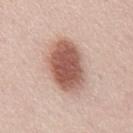– notes · catalogued during a skin exam; not biopsied
– subject · male, about 50 years old
– image-analysis metrics · an area of roughly 22 mm² and a shape-asymmetry score of about 0.1 (0 = symmetric); an average lesion color of about L≈56 a*≈22 b*≈27 (CIELAB); border irregularity of about 1.5 on a 0–10 scale, internal color variation of about 5 on a 0–10 scale, and radial color variation of about 1.5; a nevus-likeness score of about 100/100 and a detector confidence of about 100 out of 100 that the crop contains a lesion
– imaging modality · ~15 mm crop, total-body skin-cancer survey
– anatomic site · the abdomen
– illumination · white-light